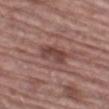Impression:
Part of a total-body skin-imaging series; this lesion was reviewed on a skin check and was not flagged for biopsy.
Background:
The tile uses white-light illumination. On the left thigh. A 15 mm crop from a total-body photograph taken for skin-cancer surveillance. A male subject in their 70s. Longest diameter approximately 4 mm.